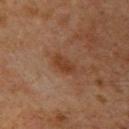Case summary:
- lighting · cross-polarized illumination
- patient · male, in their 60s
- body site · the right upper arm
- image · ~15 mm tile from a whole-body skin photo
- lesion diameter · about 3 mm